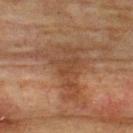{
  "patient": {
    "sex": "male",
    "age_approx": 75
  },
  "image": {
    "source": "total-body photography crop",
    "field_of_view_mm": 15
  },
  "site": "upper back"
}